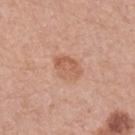Imaged during a routine full-body skin examination; the lesion was not biopsied and no histopathology is available.